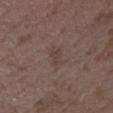Impression:
Imaged during a routine full-body skin examination; the lesion was not biopsied and no histopathology is available.
Clinical summary:
An algorithmic analysis of the crop reported an outline eccentricity of about 0.9 (0 = round, 1 = elongated) and two-axis asymmetry of about 0.4. It also reported an automated nevus-likeness rating near 0 out of 100. On the left forearm. The recorded lesion diameter is about 2.5 mm. Imaged with white-light lighting. The subject is a female roughly 35 years of age. Cropped from a whole-body photographic skin survey; the tile spans about 15 mm.